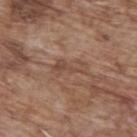Clinical impression: Part of a total-body skin-imaging series; this lesion was reviewed on a skin check and was not flagged for biopsy. Acquisition and patient details: The recorded lesion diameter is about 4 mm. From the upper back. A close-up tile cropped from a whole-body skin photograph, about 15 mm across. The subject is a male aged 68 to 72. Automated image analysis of the tile measured a footprint of about 5 mm², an eccentricity of roughly 0.9, and a symmetry-axis asymmetry near 0.55. The software also gave an average lesion color of about L≈47 a*≈19 b*≈27 (CIELAB), roughly 7 lightness units darker than nearby skin, and a normalized border contrast of about 5.5. It also reported a border-irregularity rating of about 7.5/10 and a peripheral color-asymmetry measure near 0.5. Imaged with white-light lighting.